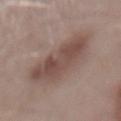Captured during whole-body skin photography for melanoma surveillance; the lesion was not biopsied.
The subject is a male about 55 years old.
The lesion is located on the mid back.
A lesion tile, about 15 mm wide, cut from a 3D total-body photograph.